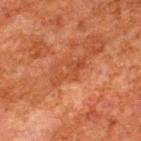Image and clinical context:
The lesion is on the upper back. The lesion's longest dimension is about 4.5 mm. Automated tile analysis of the lesion measured a shape eccentricity near 0.9 and a symmetry-axis asymmetry near 0.45. The analysis additionally found border irregularity of about 7 on a 0–10 scale. And it measured a nevus-likeness score of about 0/100. A close-up tile cropped from a whole-body skin photograph, about 15 mm across. A male subject, roughly 80 years of age.